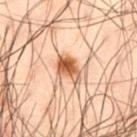| field | value |
|---|---|
| lesion size | ≈3 mm |
| image source | 15 mm crop, total-body photography |
| body site | the left thigh |
| subject | male, aged approximately 50 |
| image-analysis metrics | a mean CIELAB color near L≈45 a*≈19 b*≈30 and about 13 CIELAB-L* units darker than the surrounding skin; a color-variation rating of about 6/10 and peripheral color asymmetry of about 2 |
| illumination | cross-polarized illumination |